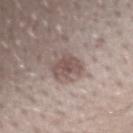Imaged during a routine full-body skin examination; the lesion was not biopsied and no histopathology is available. The lesion-visualizer software estimated a shape eccentricity near 0.55. The analysis additionally found a lesion–skin lightness drop of about 10 and a normalized lesion–skin contrast near 7. The lesion is on the right forearm. The tile uses white-light illumination. A 15 mm close-up tile from a total-body photography series done for melanoma screening. The subject is a male aged 28 to 32.